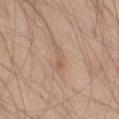The lesion was photographed on a routine skin check and not biopsied; there is no pathology result. A roughly 15 mm field-of-view crop from a total-body skin photograph. This is a white-light tile. On the left thigh. A male patient approximately 60 years of age. Automated tile analysis of the lesion measured an area of roughly 2.5 mm², a shape eccentricity near 0.95, and two-axis asymmetry of about 0.45.